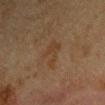Imaged during a routine full-body skin examination; the lesion was not biopsied and no histopathology is available. This image is a 15 mm lesion crop taken from a total-body photograph. The lesion is located on the arm. About 3.5 mm across. Automated image analysis of the tile measured a lesion color around L≈30 a*≈14 b*≈26 in CIELAB and a normalized lesion–skin contrast near 5.5. It also reported a classifier nevus-likeness of about 0/100. The tile uses cross-polarized illumination. A male subject, approximately 65 years of age.